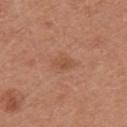lesion diameter: ~3 mm (longest diameter)
automated lesion analysis: an average lesion color of about L≈51 a*≈23 b*≈32 (CIELAB), roughly 7 lightness units darker than nearby skin, and a lesion-to-skin contrast of about 5 (normalized; higher = more distinct); a border-irregularity index near 2/10, internal color variation of about 2 on a 0–10 scale, and radial color variation of about 0.5; an automated nevus-likeness rating near 0 out of 100 and a lesion-detection confidence of about 100/100
tile lighting: white-light illumination
patient: male, aged around 30
anatomic site: the upper back
image: ~15 mm crop, total-body skin-cancer survey